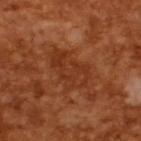Impression:
The lesion was photographed on a routine skin check and not biopsied; there is no pathology result.